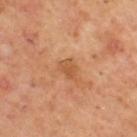Recorded during total-body skin imaging; not selected for excision or biopsy. This image is a 15 mm lesion crop taken from a total-body photograph. The lesion is located on the upper back. A male subject aged 53–57.